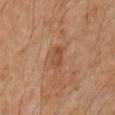| field | value |
|---|---|
| workup | total-body-photography surveillance lesion; no biopsy |
| image source | ~15 mm tile from a whole-body skin photo |
| site | the left upper arm |
| illumination | cross-polarized |
| diameter | ~3 mm (longest diameter) |
| subject | male, in their mid- to late 50s |